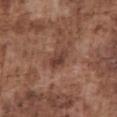notes — no biopsy performed (imaged during a skin exam)
TBP lesion metrics — a lesion area of about 4 mm², a shape eccentricity near 0.85, and a shape-asymmetry score of about 0.2 (0 = symmetric); a mean CIELAB color near L≈40 a*≈20 b*≈25, about 9 CIELAB-L* units darker than the surrounding skin, and a normalized lesion–skin contrast near 8; a border-irregularity index near 2/10, a within-lesion color-variation index near 3/10, and a peripheral color-asymmetry measure near 1; a classifier nevus-likeness of about 0/100 and a detector confidence of about 100 out of 100 that the crop contains a lesion
body site — the abdomen
patient — male, aged around 75
tile lighting — white-light illumination
imaging modality — ~15 mm crop, total-body skin-cancer survey